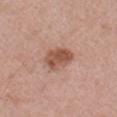No biopsy was performed on this lesion — it was imaged during a full skin examination and was not determined to be concerning. This is a white-light tile. From the right upper arm. This image is a 15 mm lesion crop taken from a total-body photograph. A female subject aged 43 to 47. The lesion-visualizer software estimated an average lesion color of about L≈53 a*≈22 b*≈29 (CIELAB) and a lesion-to-skin contrast of about 8.5 (normalized; higher = more distinct). The recorded lesion diameter is about 4 mm.